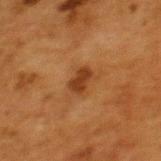{"biopsy_status": "not biopsied; imaged during a skin examination", "lesion_size": {"long_diameter_mm_approx": 2.5}, "patient": {"sex": "female", "age_approx": 50}, "site": "back", "image": {"source": "total-body photography crop", "field_of_view_mm": 15}}